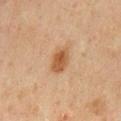{"biopsy_status": "not biopsied; imaged during a skin examination", "patient": {"sex": "male", "age_approx": 45}, "lighting": "cross-polarized", "site": "mid back", "lesion_size": {"long_diameter_mm_approx": 3.5}, "automated_metrics": {"border_irregularity_0_10": 1.5, "color_variation_0_10": 3.0, "peripheral_color_asymmetry": 1.0}, "image": {"source": "total-body photography crop", "field_of_view_mm": 15}}